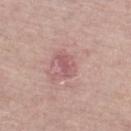Imaged during a routine full-body skin examination; the lesion was not biopsied and no histopathology is available. A female patient in their 70s. The lesion-visualizer software estimated a mean CIELAB color near L≈57 a*≈24 b*≈20, roughly 9 lightness units darker than nearby skin, and a normalized border contrast of about 6. The software also gave a classifier nevus-likeness of about 0/100 and a lesion-detection confidence of about 100/100. A 15 mm close-up extracted from a 3D total-body photography capture. This is a white-light tile. The lesion is located on the left lower leg. Longest diameter approximately 3 mm.